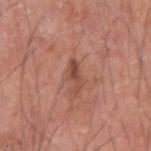Case summary:
* follow-up · imaged on a skin check; not biopsied
* lighting · white-light illumination
* subject · male, about 75 years old
* location · the right upper arm
* lesion diameter · ≈4 mm
* acquisition · ~15 mm crop, total-body skin-cancer survey
* image-analysis metrics · an area of roughly 4 mm²